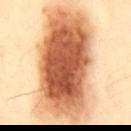Imaged during a routine full-body skin examination; the lesion was not biopsied and no histopathology is available.
Located on the chest.
A lesion tile, about 15 mm wide, cut from a 3D total-body photograph.
A male patient, aged approximately 50.